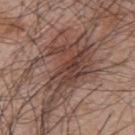Assessment:
Captured during whole-body skin photography for melanoma surveillance; the lesion was not biopsied.
Context:
On the upper back. The patient is a male aged 48–52. A close-up tile cropped from a whole-body skin photograph, about 15 mm across.